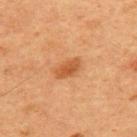Impression: Part of a total-body skin-imaging series; this lesion was reviewed on a skin check and was not flagged for biopsy. Background: A male patient aged around 60. Imaged with cross-polarized lighting. From the chest. Cropped from a whole-body photographic skin survey; the tile spans about 15 mm.